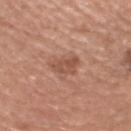Impression:
Imaged during a routine full-body skin examination; the lesion was not biopsied and no histopathology is available.
Acquisition and patient details:
A male patient, aged approximately 75. A region of skin cropped from a whole-body photographic capture, roughly 15 mm wide. The tile uses white-light illumination. The recorded lesion diameter is about 3.5 mm. The lesion is on the head or neck.